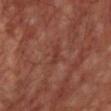workup = catalogued during a skin exam; not biopsied
lesion diameter = about 2.5 mm
image = 15 mm crop, total-body photography
location = the chest
subject = male, aged 53 to 57